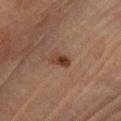Case summary:
- biopsy status · imaged on a skin check; not biopsied